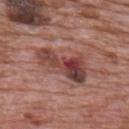Captured during whole-body skin photography for melanoma surveillance; the lesion was not biopsied.
A region of skin cropped from a whole-body photographic capture, roughly 15 mm wide.
A male patient aged 68 to 72.
Approximately 6.5 mm at its widest.
Located on the back.